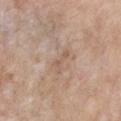The lesion is located on the front of the torso. A female patient aged 73 to 77. The recorded lesion diameter is about 3 mm. Cropped from a whole-body photographic skin survey; the tile spans about 15 mm. This is a white-light tile.